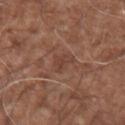Assessment:
Imaged during a routine full-body skin examination; the lesion was not biopsied and no histopathology is available.
Acquisition and patient details:
Measured at roughly 3 mm in maximum diameter. The lesion is located on the arm. The tile uses white-light illumination. A lesion tile, about 15 mm wide, cut from a 3D total-body photograph. A male subject about 75 years old. Automated image analysis of the tile measured a lesion–skin lightness drop of about 6 and a normalized lesion–skin contrast near 5. And it measured a border-irregularity rating of about 4/10, internal color variation of about 2.5 on a 0–10 scale, and radial color variation of about 1.